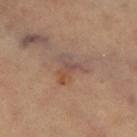No biopsy was performed on this lesion — it was imaged during a full skin examination and was not determined to be concerning.
Located on the leg.
The subject is a female roughly 70 years of age.
A close-up tile cropped from a whole-body skin photograph, about 15 mm across.